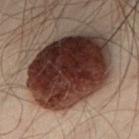Background:
The tile uses cross-polarized illumination. A male subject about 50 years old. The lesion is on the front of the torso. Approximately 10 mm at its widest. A roughly 15 mm field-of-view crop from a total-body skin photograph. An algorithmic analysis of the crop reported an area of roughly 60 mm², an outline eccentricity of about 0.7 (0 = round, 1 = elongated), and a symmetry-axis asymmetry near 0.1.